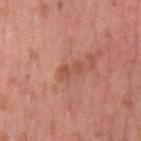Impression:
Recorded during total-body skin imaging; not selected for excision or biopsy.
Context:
A male subject roughly 55 years of age. This is a white-light tile. A 15 mm close-up extracted from a 3D total-body photography capture. An algorithmic analysis of the crop reported an area of roughly 3 mm², an eccentricity of roughly 0.9, and a shape-asymmetry score of about 0.3 (0 = symmetric). The analysis additionally found a lesion color around L≈52 a*≈26 b*≈31 in CIELAB. The analysis additionally found a border-irregularity index near 5/10, internal color variation of about 0.5 on a 0–10 scale, and a peripheral color-asymmetry measure near 0.5. On the left upper arm.